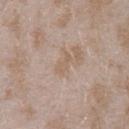Case summary:
- follow-up: imaged on a skin check; not biopsied
- illumination: white-light illumination
- patient: female, in their mid-20s
- image source: ~15 mm tile from a whole-body skin photo
- site: the left forearm
- TBP lesion metrics: a lesion color around L≈59 a*≈15 b*≈28 in CIELAB and a lesion-to-skin contrast of about 5 (normalized; higher = more distinct); a classifier nevus-likeness of about 0/100 and lesion-presence confidence of about 95/100